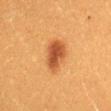Impression: Imaged during a routine full-body skin examination; the lesion was not biopsied and no histopathology is available. Image and clinical context: The patient is a female approximately 40 years of age. On the mid back. The lesion-visualizer software estimated a footprint of about 10 mm², an outline eccentricity of about 0.75 (0 = round, 1 = elongated), and two-axis asymmetry of about 0.2. The software also gave roughly 12 lightness units darker than nearby skin and a lesion-to-skin contrast of about 9 (normalized; higher = more distinct). The software also gave a classifier nevus-likeness of about 100/100 and lesion-presence confidence of about 100/100. The lesion's longest dimension is about 4 mm. Captured under cross-polarized illumination. A roughly 15 mm field-of-view crop from a total-body skin photograph.